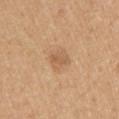No biopsy was performed on this lesion — it was imaged during a full skin examination and was not determined to be concerning.